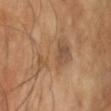Imaged during a routine full-body skin examination; the lesion was not biopsied and no histopathology is available.
The lesion is located on the head or neck.
The patient is a male aged 43–47.
Cropped from a total-body skin-imaging series; the visible field is about 15 mm.